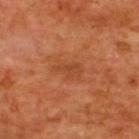* follow-up · no biopsy performed (imaged during a skin exam)
* patient · male, aged approximately 65
* location · the upper back
* illumination · cross-polarized illumination
* imaging modality · ~15 mm crop, total-body skin-cancer survey